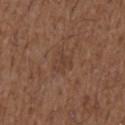Part of a total-body skin-imaging series; this lesion was reviewed on a skin check and was not flagged for biopsy.
On the right upper arm.
This is a white-light tile.
A roughly 15 mm field-of-view crop from a total-body skin photograph.
About 2.5 mm across.
A male patient, aged 48 to 52.